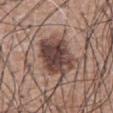Acquisition and patient details: A male subject, about 60 years old. On the chest. A region of skin cropped from a whole-body photographic capture, roughly 15 mm wide.